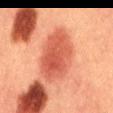Impression: The lesion was photographed on a routine skin check and not biopsied; there is no pathology result. Background: The lesion is on the mid back. The subject is a male in their 40s. Imaged with cross-polarized lighting. The total-body-photography lesion software estimated an outline eccentricity of about 0.8 (0 = round, 1 = elongated) and two-axis asymmetry of about 0.1. It also reported border irregularity of about 1.5 on a 0–10 scale, a within-lesion color-variation index near 4/10, and a peripheral color-asymmetry measure near 1. Cropped from a total-body skin-imaging series; the visible field is about 15 mm.